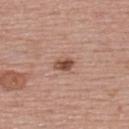No biopsy was performed on this lesion — it was imaged during a full skin examination and was not determined to be concerning.
Located on the back.
Measured at roughly 2.5 mm in maximum diameter.
A roughly 15 mm field-of-view crop from a total-body skin photograph.
Captured under white-light illumination.
A female subject, aged approximately 55.